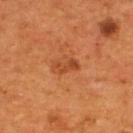No biopsy was performed on this lesion — it was imaged during a full skin examination and was not determined to be concerning. From the back. A male subject roughly 55 years of age. A 15 mm crop from a total-body photograph taken for skin-cancer surveillance. The lesion's longest dimension is about 3 mm.